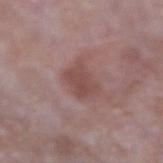Clinical impression:
No biopsy was performed on this lesion — it was imaged during a full skin examination and was not determined to be concerning.
Acquisition and patient details:
A 15 mm close-up extracted from a 3D total-body photography capture. The patient is a male in their mid- to late 60s. The lesion is located on the left forearm.